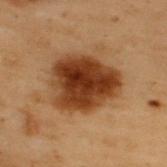Case summary:
* follow-up — no biopsy performed (imaged during a skin exam)
* acquisition — total-body-photography crop, ~15 mm field of view
* anatomic site — the back
* lesion size — ~6.5 mm (longest diameter)
* patient — male, in their mid-50s
* lighting — cross-polarized